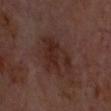This lesion was catalogued during total-body skin photography and was not selected for biopsy.
This is a cross-polarized tile.
A subject in their mid-60s.
A roughly 15 mm field-of-view crop from a total-body skin photograph.
Located on the head or neck.
The lesion's longest dimension is about 4.5 mm.